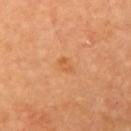Q: Was a biopsy performed?
A: imaged on a skin check; not biopsied
Q: What did automated image analysis measure?
A: a footprint of about 3 mm², an outline eccentricity of about 0.8 (0 = round, 1 = elongated), and two-axis asymmetry of about 0.25; an average lesion color of about L≈61 a*≈27 b*≈44 (CIELAB), roughly 6 lightness units darker than nearby skin, and a normalized lesion–skin contrast near 5; a nevus-likeness score of about 0/100 and lesion-presence confidence of about 100/100
Q: What is the anatomic site?
A: the left upper arm
Q: Who is the patient?
A: female, approximately 60 years of age
Q: What kind of image is this?
A: ~15 mm crop, total-body skin-cancer survey
Q: What lighting was used for the tile?
A: cross-polarized